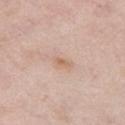biopsy status=catalogued during a skin exam; not biopsied
image source=~15 mm tile from a whole-body skin photo
subject=female, in their mid- to late 60s
anatomic site=the chest